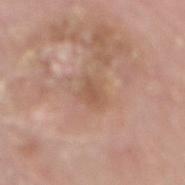Q: Was a biopsy performed?
A: catalogued during a skin exam; not biopsied
Q: What is the imaging modality?
A: ~15 mm tile from a whole-body skin photo
Q: Who is the patient?
A: female, aged approximately 65
Q: What is the anatomic site?
A: the chest
Q: How large is the lesion?
A: about 3 mm